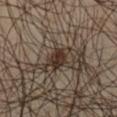Recorded during total-body skin imaging; not selected for excision or biopsy. The lesion is located on the left thigh. A region of skin cropped from a whole-body photographic capture, roughly 15 mm wide. Automated image analysis of the tile measured an average lesion color of about L≈23 a*≈10 b*≈17 (CIELAB), about 8 CIELAB-L* units darker than the surrounding skin, and a normalized lesion–skin contrast near 10. And it measured a nevus-likeness score of about 100/100 and a detector confidence of about 95 out of 100 that the crop contains a lesion. A male patient approximately 50 years of age.